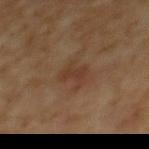{
  "biopsy_status": "not biopsied; imaged during a skin examination",
  "patient": {
    "sex": "male",
    "age_approx": 60
  },
  "site": "mid back",
  "lesion_size": {
    "long_diameter_mm_approx": 3.0
  },
  "lighting": "cross-polarized",
  "image": {
    "source": "total-body photography crop",
    "field_of_view_mm": 15
  }
}